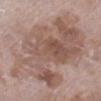Imaged during a routine full-body skin examination; the lesion was not biopsied and no histopathology is available. The lesion is located on the left lower leg. The subject is a female in their 70s. Measured at roughly 13.5 mm in maximum diameter. Captured under white-light illumination. A 15 mm close-up tile from a total-body photography series done for melanoma screening.